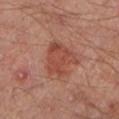Assessment: Imaged during a routine full-body skin examination; the lesion was not biopsied and no histopathology is available. Clinical summary: The patient is a male roughly 70 years of age. From the left lower leg. A 15 mm close-up extracted from a 3D total-body photography capture.